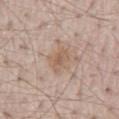No biopsy was performed on this lesion — it was imaged during a full skin examination and was not determined to be concerning.
Located on the lower back.
A lesion tile, about 15 mm wide, cut from a 3D total-body photograph.
A male subject in their mid- to late 60s.
The tile uses white-light illumination.